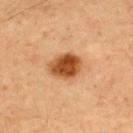Clinical impression: The lesion was photographed on a routine skin check and not biopsied; there is no pathology result. Clinical summary: Imaged with cross-polarized lighting. The subject is a male aged 48 to 52. The lesion is located on the upper back. A close-up tile cropped from a whole-body skin photograph, about 15 mm across. The recorded lesion diameter is about 4 mm.